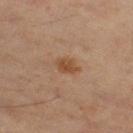Impression: Captured during whole-body skin photography for melanoma surveillance; the lesion was not biopsied. Background: Imaged with cross-polarized lighting. Cropped from a whole-body photographic skin survey; the tile spans about 15 mm. The total-body-photography lesion software estimated a lesion area of about 4.5 mm² and an eccentricity of roughly 0.8. The software also gave a lesion color around L≈44 a*≈19 b*≈31 in CIELAB and a lesion-to-skin contrast of about 7.5 (normalized; higher = more distinct). It also reported a border-irregularity index near 2/10, a within-lesion color-variation index near 1.5/10, and a peripheral color-asymmetry measure near 0.5. A male patient aged approximately 60. Located on the right thigh. Longest diameter approximately 3 mm.